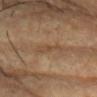Impression:
Imaged during a routine full-body skin examination; the lesion was not biopsied and no histopathology is available.
Context:
Captured under cross-polarized illumination. A female subject approximately 80 years of age. The recorded lesion diameter is about 4 mm. On the chest. A roughly 15 mm field-of-view crop from a total-body skin photograph. The total-body-photography lesion software estimated a lesion area of about 5 mm², an eccentricity of roughly 0.95, and a shape-asymmetry score of about 0.35 (0 = symmetric).